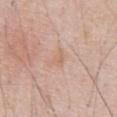This lesion was catalogued during total-body skin photography and was not selected for biopsy.
The tile uses white-light illumination.
A male patient, approximately 70 years of age.
The lesion is on the chest.
A close-up tile cropped from a whole-body skin photograph, about 15 mm across.